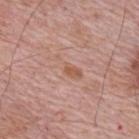This lesion was catalogued during total-body skin photography and was not selected for biopsy. The subject is a male about 65 years old. This is a white-light tile. A roughly 15 mm field-of-view crop from a total-body skin photograph. On the upper back.